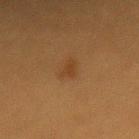Assessment: Part of a total-body skin-imaging series; this lesion was reviewed on a skin check and was not flagged for biopsy. Background: This image is a 15 mm lesion crop taken from a total-body photograph. From the back. A female patient, roughly 40 years of age.